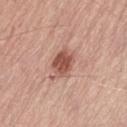Notes:
• follow-up — imaged on a skin check; not biopsied
• anatomic site — the left thigh
• acquisition — ~15 mm tile from a whole-body skin photo
• lesion size — about 3.5 mm
• patient — male, in their mid-70s